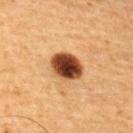The patient is a male aged 63 to 67.
A 15 mm close-up extracted from a 3D total-body photography capture.
The lesion is on the upper back.